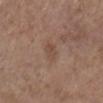Q: Was this lesion biopsied?
A: catalogued during a skin exam; not biopsied
Q: Where on the body is the lesion?
A: the left lower leg
Q: Automated lesion metrics?
A: a lesion area of about 4 mm², an outline eccentricity of about 0.7 (0 = round, 1 = elongated), and a shape-asymmetry score of about 0.2 (0 = symmetric); an average lesion color of about L≈47 a*≈17 b*≈27 (CIELAB), roughly 6 lightness units darker than nearby skin, and a normalized border contrast of about 5; border irregularity of about 2 on a 0–10 scale, internal color variation of about 3 on a 0–10 scale, and peripheral color asymmetry of about 1; a classifier nevus-likeness of about 0/100 and lesion-presence confidence of about 100/100
Q: What kind of image is this?
A: total-body-photography crop, ~15 mm field of view
Q: Illumination type?
A: white-light illumination
Q: What are the patient's age and sex?
A: female, approximately 65 years of age
Q: How large is the lesion?
A: about 2.5 mm